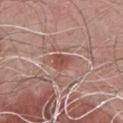Clinical impression:
The lesion was photographed on a routine skin check and not biopsied; there is no pathology result.
Clinical summary:
This image is a 15 mm lesion crop taken from a total-body photograph. A male subject aged approximately 60. The tile uses white-light illumination. On the chest.